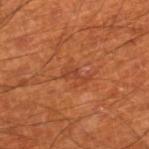{"biopsy_status": "not biopsied; imaged during a skin examination", "lesion_size": {"long_diameter_mm_approx": 3.0}, "site": "right thigh", "image": {"source": "total-body photography crop", "field_of_view_mm": 15}, "lighting": "cross-polarized", "patient": {"sex": "male", "age_approx": 70}, "automated_metrics": {"eccentricity": 0.9, "cielab_L": 42, "cielab_a": 29, "cielab_b": 35, "vs_skin_darker_L": 6.0, "vs_skin_contrast_norm": 5.5, "nevus_likeness_0_100": 0, "lesion_detection_confidence_0_100": 100}}